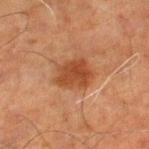Q: Was a biopsy performed?
A: imaged on a skin check; not biopsied
Q: What kind of image is this?
A: 15 mm crop, total-body photography
Q: What are the patient's age and sex?
A: male, in their 70s
Q: Automated lesion metrics?
A: an area of roughly 11 mm², a shape eccentricity near 0.6, and two-axis asymmetry of about 0.2; an average lesion color of about L≈39 a*≈23 b*≈32 (CIELAB), a lesion–skin lightness drop of about 9, and a lesion-to-skin contrast of about 8 (normalized; higher = more distinct); border irregularity of about 2.5 on a 0–10 scale, a color-variation rating of about 3/10, and a peripheral color-asymmetry measure near 1; an automated nevus-likeness rating near 80 out of 100 and lesion-presence confidence of about 100/100
Q: Lesion location?
A: the left lower leg
Q: What lighting was used for the tile?
A: cross-polarized
Q: What is the lesion's diameter?
A: ≈4.5 mm